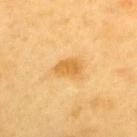No biopsy was performed on this lesion — it was imaged during a full skin examination and was not determined to be concerning. On the upper back. Approximately 3 mm at its widest. A region of skin cropped from a whole-body photographic capture, roughly 15 mm wide. A male patient, approximately 60 years of age.